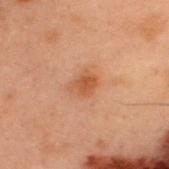Clinical impression:
The lesion was photographed on a routine skin check and not biopsied; there is no pathology result.